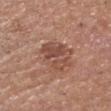Impression: This lesion was catalogued during total-body skin photography and was not selected for biopsy. Clinical summary: Automated image analysis of the tile measured an average lesion color of about L≈48 a*≈22 b*≈27 (CIELAB), a lesion–skin lightness drop of about 9, and a normalized lesion–skin contrast near 6.5. It also reported a border-irregularity index near 5.5/10, a color-variation rating of about 5/10, and peripheral color asymmetry of about 2. And it measured a nevus-likeness score of about 0/100 and lesion-presence confidence of about 100/100. A male patient, aged approximately 65. Captured under white-light illumination. The lesion is on the head or neck. The recorded lesion diameter is about 4 mm. A lesion tile, about 15 mm wide, cut from a 3D total-body photograph.